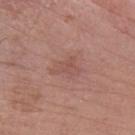This lesion was catalogued during total-body skin photography and was not selected for biopsy.
Automated image analysis of the tile measured a within-lesion color-variation index near 1.5/10 and peripheral color asymmetry of about 0.5.
A 15 mm crop from a total-body photograph taken for skin-cancer surveillance.
A male subject aged 53–57.
This is a white-light tile.
Longest diameter approximately 3.5 mm.
On the left forearm.